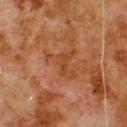Case summary:
– workup · catalogued during a skin exam; not biopsied
– acquisition · 15 mm crop, total-body photography
– location · the upper back
– patient · male, roughly 80 years of age